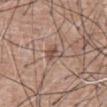No biopsy was performed on this lesion — it was imaged during a full skin examination and was not determined to be concerning. An algorithmic analysis of the crop reported border irregularity of about 3.5 on a 0–10 scale and a peripheral color-asymmetry measure near 1.5. This image is a 15 mm lesion crop taken from a total-body photograph. The subject is a male aged approximately 60. The lesion is on the abdomen.